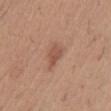The lesion was photographed on a routine skin check and not biopsied; there is no pathology result.
The patient is a male about 60 years old.
The lesion is on the abdomen.
Approximately 2.5 mm at its widest.
This image is a 15 mm lesion crop taken from a total-body photograph.